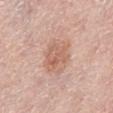Impression:
Part of a total-body skin-imaging series; this lesion was reviewed on a skin check and was not flagged for biopsy.
Acquisition and patient details:
An algorithmic analysis of the crop reported a footprint of about 13 mm², an outline eccentricity of about 0.6 (0 = round, 1 = elongated), and a shape-asymmetry score of about 0.2 (0 = symmetric). The analysis additionally found an average lesion color of about L≈62 a*≈21 b*≈29 (CIELAB), about 9 CIELAB-L* units darker than the surrounding skin, and a normalized lesion–skin contrast near 6. Measured at roughly 4.5 mm in maximum diameter. A region of skin cropped from a whole-body photographic capture, roughly 15 mm wide. Located on the left lower leg. The tile uses white-light illumination. A female subject, in their mid- to late 60s.